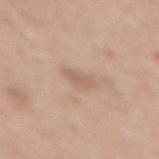notes: catalogued during a skin exam; not biopsied | tile lighting: white-light illumination | lesion diameter: ~3 mm (longest diameter) | location: the mid back | patient: female, aged 48–52 | image: ~15 mm crop, total-body skin-cancer survey.